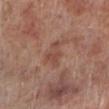Part of a total-body skin-imaging series; this lesion was reviewed on a skin check and was not flagged for biopsy. The lesion is on the left lower leg. The recorded lesion diameter is about 3.5 mm. Captured under white-light illumination. A lesion tile, about 15 mm wide, cut from a 3D total-body photograph. A male patient aged approximately 70.